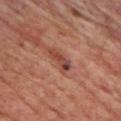workup: catalogued during a skin exam; not biopsied
body site: the chest
patient: male, aged 63 to 67
tile lighting: cross-polarized illumination
lesion size: ~3.5 mm (longest diameter)
imaging modality: ~15 mm crop, total-body skin-cancer survey